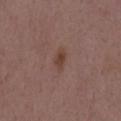Captured during whole-body skin photography for melanoma surveillance; the lesion was not biopsied. A male subject, aged 48–52. Located on the mid back. This is a white-light tile. A lesion tile, about 15 mm wide, cut from a 3D total-body photograph. Approximately 3 mm at its widest. An algorithmic analysis of the crop reported an area of roughly 3.5 mm², an outline eccentricity of about 0.9 (0 = round, 1 = elongated), and a shape-asymmetry score of about 0.3 (0 = symmetric). And it measured about 7 CIELAB-L* units darker than the surrounding skin and a normalized lesion–skin contrast near 7. It also reported an automated nevus-likeness rating near 35 out of 100 and a lesion-detection confidence of about 100/100.